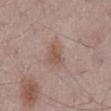Recorded during total-body skin imaging; not selected for excision or biopsy. Cropped from a total-body skin-imaging series; the visible field is about 15 mm. A male patient about 65 years old. Imaged with white-light lighting. From the abdomen.